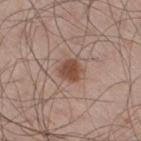Clinical impression: The lesion was photographed on a routine skin check and not biopsied; there is no pathology result. Clinical summary: Longest diameter approximately 2.5 mm. A male patient roughly 60 years of age. From the leg. Imaged with white-light lighting. Automated tile analysis of the lesion measured an outline eccentricity of about 0.25 (0 = round, 1 = elongated) and two-axis asymmetry of about 0.25. And it measured a lesion color around L≈47 a*≈20 b*≈27 in CIELAB, a lesion–skin lightness drop of about 12, and a lesion-to-skin contrast of about 9 (normalized; higher = more distinct). It also reported border irregularity of about 2 on a 0–10 scale, a within-lesion color-variation index near 3/10, and peripheral color asymmetry of about 1. It also reported a nevus-likeness score of about 95/100 and a detector confidence of about 100 out of 100 that the crop contains a lesion. A lesion tile, about 15 mm wide, cut from a 3D total-body photograph.